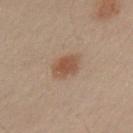This lesion was catalogued during total-body skin photography and was not selected for biopsy.
This is a cross-polarized tile.
The lesion's longest dimension is about 3 mm.
On the left upper arm.
The total-body-photography lesion software estimated an eccentricity of roughly 0.5 and a symmetry-axis asymmetry near 0.2.
A male patient, roughly 50 years of age.
A 15 mm crop from a total-body photograph taken for skin-cancer surveillance.